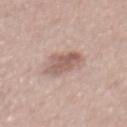biopsy status — total-body-photography surveillance lesion; no biopsy
image — 15 mm crop, total-body photography
lesion size — ≈4.5 mm
lighting — white-light illumination
patient — male, aged approximately 55
location — the back
TBP lesion metrics — a lesion area of about 9 mm² and an outline eccentricity of about 0.8 (0 = round, 1 = elongated); about 11 CIELAB-L* units darker than the surrounding skin; a border-irregularity index near 3/10 and a peripheral color-asymmetry measure near 1.5; lesion-presence confidence of about 100/100